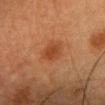<case>
  <biopsy_status>not biopsied; imaged during a skin examination</biopsy_status>
</case>